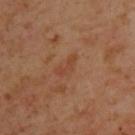Case summary:
- notes — catalogued during a skin exam; not biopsied
- tile lighting — cross-polarized
- size — ≈3 mm
- image-analysis metrics — a lesion area of about 3 mm², a shape eccentricity near 0.9, and a shape-asymmetry score of about 0.55 (0 = symmetric); a lesion color around L≈43 a*≈24 b*≈32 in CIELAB, a lesion–skin lightness drop of about 5, and a normalized border contrast of about 5; a nevus-likeness score of about 0/100 and lesion-presence confidence of about 100/100
- location — the upper back
- image — ~15 mm tile from a whole-body skin photo
- patient — male, aged approximately 45